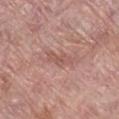location: the right lower leg | lesion size: ~3.5 mm (longest diameter) | patient: female, aged around 60 | image source: ~15 mm tile from a whole-body skin photo | automated metrics: a detector confidence of about 95 out of 100 that the crop contains a lesion.